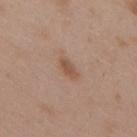This lesion was catalogued during total-body skin photography and was not selected for biopsy. A male subject aged 48 to 52. Automated image analysis of the tile measured a lesion area of about 3 mm², an eccentricity of roughly 0.9, and a symmetry-axis asymmetry near 0.35. And it measured a lesion color around L≈52 a*≈19 b*≈30 in CIELAB, a lesion–skin lightness drop of about 9, and a lesion-to-skin contrast of about 7 (normalized; higher = more distinct). The analysis additionally found a border-irregularity rating of about 3/10, a within-lesion color-variation index near 1/10, and radial color variation of about 0.5. And it measured a classifier nevus-likeness of about 70/100 and a lesion-detection confidence of about 100/100. A 15 mm close-up tile from a total-body photography series done for melanoma screening. The tile uses white-light illumination. From the upper back.